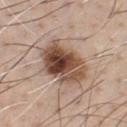Clinical impression: The lesion was tiled from a total-body skin photograph and was not biopsied.